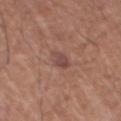| feature | finding |
|---|---|
| workup | total-body-photography surveillance lesion; no biopsy |
| diameter | ~3.5 mm (longest diameter) |
| subject | male, in their mid-60s |
| location | the chest |
| tile lighting | white-light illumination |
| image | total-body-photography crop, ~15 mm field of view |